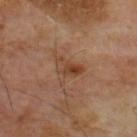Case summary:
- notes — no biopsy performed (imaged during a skin exam)
- subject — male, aged approximately 65
- tile lighting — cross-polarized
- anatomic site — the back
- image-analysis metrics — an area of roughly 5 mm², an outline eccentricity of about 0.85 (0 = round, 1 = elongated), and a shape-asymmetry score of about 0.35 (0 = symmetric); a border-irregularity rating of about 3.5/10, internal color variation of about 7.5 on a 0–10 scale, and radial color variation of about 2.5
- acquisition — total-body-photography crop, ~15 mm field of view
- lesion size — about 3.5 mm